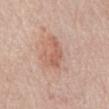Captured during whole-body skin photography for melanoma surveillance; the lesion was not biopsied.
The patient is a female aged 63–67.
This image is a 15 mm lesion crop taken from a total-body photograph.
The lesion's longest dimension is about 4.5 mm.
This is a white-light tile.
The lesion is located on the chest.